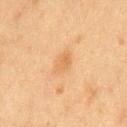Q: Is there a histopathology result?
A: imaged on a skin check; not biopsied
Q: What are the patient's age and sex?
A: male, aged 73–77
Q: How was this image acquired?
A: ~15 mm tile from a whole-body skin photo
Q: How large is the lesion?
A: ~3.5 mm (longest diameter)
Q: Where on the body is the lesion?
A: the chest
Q: What lighting was used for the tile?
A: cross-polarized